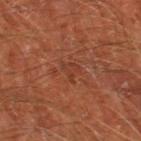biopsy_status: not biopsied; imaged during a skin examination
image:
  source: total-body photography crop
  field_of_view_mm: 15
automated_metrics:
  cielab_L: 37
  cielab_a: 25
  cielab_b: 30
  vs_skin_darker_L: 5.0
  vs_skin_contrast_norm: 4.5
  border_irregularity_0_10: 5.0
  color_variation_0_10: 2.0
lighting: cross-polarized
site: left thigh
lesion_size:
  long_diameter_mm_approx: 2.5
patient:
  sex: male
  age_approx: 65